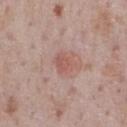The lesion was tiled from a total-body skin photograph and was not biopsied.
The recorded lesion diameter is about 2.5 mm.
This image is a 15 mm lesion crop taken from a total-body photograph.
The lesion-visualizer software estimated a lesion-detection confidence of about 100/100.
A male patient approximately 75 years of age.
The lesion is located on the chest.